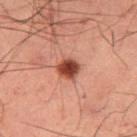Q: Was this lesion biopsied?
A: total-body-photography surveillance lesion; no biopsy
Q: What is the imaging modality?
A: ~15 mm tile from a whole-body skin photo
Q: How large is the lesion?
A: ~2.5 mm (longest diameter)
Q: What is the anatomic site?
A: the right thigh
Q: Illumination type?
A: cross-polarized
Q: Who is the patient?
A: male, aged 58–62